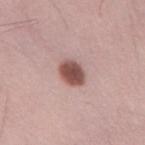{"image": {"source": "total-body photography crop", "field_of_view_mm": 15}, "automated_metrics": {"area_mm2_approx": 6.5, "eccentricity": 0.75, "shape_asymmetry": 0.15, "cielab_L": 51, "cielab_a": 21, "cielab_b": 23, "vs_skin_contrast_norm": 11.5, "border_irregularity_0_10": 1.5, "color_variation_0_10": 2.5, "peripheral_color_asymmetry": 1.0, "nevus_likeness_0_100": 100, "lesion_detection_confidence_0_100": 100}, "lesion_size": {"long_diameter_mm_approx": 3.5}, "patient": {"sex": "male", "age_approx": 35}, "lighting": "white-light", "site": "back"}